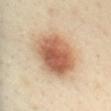Q: Was a biopsy performed?
A: imaged on a skin check; not biopsied
Q: How large is the lesion?
A: ≈6 mm
Q: What is the imaging modality?
A: 15 mm crop, total-body photography
Q: Patient demographics?
A: female, aged around 40
Q: Where on the body is the lesion?
A: the back